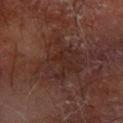{"biopsy_status": "not biopsied; imaged during a skin examination", "image": {"source": "total-body photography crop", "field_of_view_mm": 15}, "lesion_size": {"long_diameter_mm_approx": 6.0}, "patient": {"sex": "male", "age_approx": 70}, "lighting": "cross-polarized", "site": "left forearm", "automated_metrics": {"area_mm2_approx": 22.0, "eccentricity": 0.3, "shape_asymmetry": 0.4, "border_irregularity_0_10": 4.5, "peripheral_color_asymmetry": 1.0, "nevus_likeness_0_100": 0, "lesion_detection_confidence_0_100": 95}}